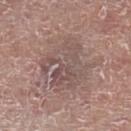{
  "biopsy_status": "not biopsied; imaged during a skin examination",
  "lighting": "white-light",
  "site": "left lower leg",
  "patient": {
    "sex": "male",
    "age_approx": 80
  },
  "image": {
    "source": "total-body photography crop",
    "field_of_view_mm": 15
  }
}